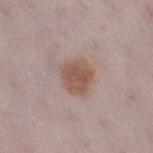This lesion was catalogued during total-body skin photography and was not selected for biopsy.
Automated image analysis of the tile measured a lesion area of about 9.5 mm². It also reported a border-irregularity index near 2/10, a within-lesion color-variation index near 3/10, and peripheral color asymmetry of about 1.
The lesion is located on the leg.
About 3.5 mm across.
A roughly 15 mm field-of-view crop from a total-body skin photograph.
The tile uses white-light illumination.
A female subject, aged 28–32.